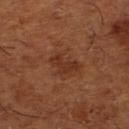Clinical summary:
The lesion is located on the right lower leg. Captured under cross-polarized illumination. A male patient in their mid-60s. A region of skin cropped from a whole-body photographic capture, roughly 15 mm wide.